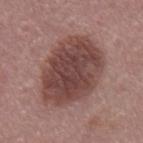follow-up = imaged on a skin check; not biopsied
acquisition = ~15 mm crop, total-body skin-cancer survey
patient = male, aged around 55
site = the mid back
lesion diameter = ~8.5 mm (longest diameter)
illumination = white-light